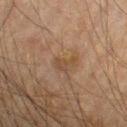Captured during whole-body skin photography for melanoma surveillance; the lesion was not biopsied.
The recorded lesion diameter is about 2.5 mm.
The tile uses cross-polarized illumination.
The total-body-photography lesion software estimated a lesion area of about 3 mm², an outline eccentricity of about 0.8 (0 = round, 1 = elongated), and two-axis asymmetry of about 0.65. And it measured a mean CIELAB color near L≈36 a*≈14 b*≈26 and roughly 6 lightness units darker than nearby skin. The software also gave a border-irregularity rating of about 7/10 and a peripheral color-asymmetry measure near 0.5.
A male subject, roughly 65 years of age.
The lesion is located on the left upper arm.
A 15 mm close-up tile from a total-body photography series done for melanoma screening.